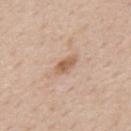Impression: The lesion was tiled from a total-body skin photograph and was not biopsied. Acquisition and patient details: On the mid back. Captured under white-light illumination. A 15 mm crop from a total-body photograph taken for skin-cancer surveillance. Measured at roughly 3 mm in maximum diameter. A male subject about 60 years old. Automated tile analysis of the lesion measured a footprint of about 3.5 mm², a shape eccentricity near 0.85, and a symmetry-axis asymmetry near 0.25. And it measured about 11 CIELAB-L* units darker than the surrounding skin and a normalized lesion–skin contrast near 7.5. It also reported border irregularity of about 2.5 on a 0–10 scale and radial color variation of about 1.